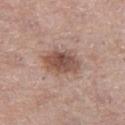follow-up=total-body-photography surveillance lesion; no biopsy
image=total-body-photography crop, ~15 mm field of view
location=the right thigh
patient=female, in their mid-60s